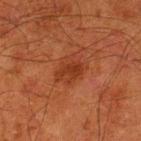Case summary:
– follow-up · total-body-photography surveillance lesion; no biopsy
– anatomic site · the left lower leg
– size · ≈3.5 mm
– patient · male, aged approximately 80
– image source · ~15 mm tile from a whole-body skin photo
– lighting · cross-polarized